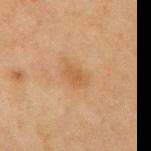<tbp_lesion>
<biopsy_status>not biopsied; imaged during a skin examination</biopsy_status>
<lesion_size>
  <long_diameter_mm_approx>2.5</long_diameter_mm_approx>
</lesion_size>
<site>left upper arm</site>
<automated_metrics>
  <eccentricity>0.8</eccentricity>
  <shape_asymmetry>0.2</shape_asymmetry>
</automated_metrics>
<image>
  <source>total-body photography crop</source>
  <field_of_view_mm>15</field_of_view_mm>
</image>
<patient>
  <sex>male</sex>
  <age_approx>65</age_approx>
</patient>
</tbp_lesion>